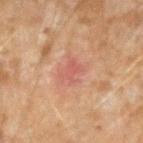Notes:
– subject · male, approximately 45 years of age
– lighting · cross-polarized
– size · ≈3 mm
– image · ~15 mm crop, total-body skin-cancer survey
– location · the left forearm